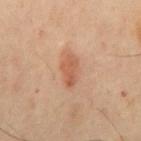Recorded during total-body skin imaging; not selected for excision or biopsy. The lesion's longest dimension is about 3.5 mm. A male subject about 45 years old. A region of skin cropped from a whole-body photographic capture, roughly 15 mm wide. The lesion is on the back. Captured under cross-polarized illumination.